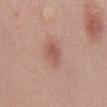Imaged during a routine full-body skin examination; the lesion was not biopsied and no histopathology is available.
The subject is a male in their mid-30s.
From the mid back.
About 3.5 mm across.
A 15 mm close-up tile from a total-body photography series done for melanoma screening.